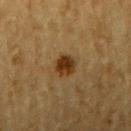notes: total-body-photography surveillance lesion; no biopsy
site: the right upper arm
image source: ~15 mm crop, total-body skin-cancer survey
illumination: cross-polarized illumination
lesion diameter: about 2.5 mm
subject: male, in their mid- to late 80s or older